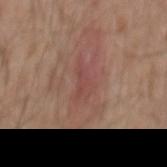Q: Is there a histopathology result?
A: total-body-photography surveillance lesion; no biopsy
Q: Lesion location?
A: the mid back
Q: Illumination type?
A: white-light illumination
Q: Patient demographics?
A: male, aged around 55
Q: Automated lesion metrics?
A: an outline eccentricity of about 0.9 (0 = round, 1 = elongated) and two-axis asymmetry of about 0.45; a border-irregularity rating of about 5/10, internal color variation of about 2 on a 0–10 scale, and a peripheral color-asymmetry measure near 0.5; a classifier nevus-likeness of about 0/100 and a detector confidence of about 85 out of 100 that the crop contains a lesion
Q: What is the imaging modality?
A: 15 mm crop, total-body photography